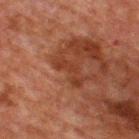Q: Is there a histopathology result?
A: no biopsy performed (imaged during a skin exam)
Q: How was the tile lit?
A: cross-polarized illumination
Q: What are the patient's age and sex?
A: male, roughly 60 years of age
Q: What is the anatomic site?
A: the upper back
Q: What is the imaging modality?
A: total-body-photography crop, ~15 mm field of view
Q: How large is the lesion?
A: ~4 mm (longest diameter)